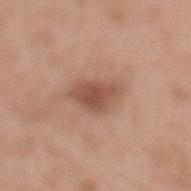{
  "biopsy_status": "not biopsied; imaged during a skin examination",
  "lesion_size": {
    "long_diameter_mm_approx": 4.5
  },
  "image": {
    "source": "total-body photography crop",
    "field_of_view_mm": 15
  },
  "patient": {
    "sex": "female",
    "age_approx": 70
  },
  "lighting": "white-light",
  "site": "mid back",
  "automated_metrics": {
    "cielab_L": 52,
    "cielab_a": 21,
    "cielab_b": 29,
    "vs_skin_darker_L": 11.0,
    "vs_skin_contrast_norm": 7.5,
    "nevus_likeness_0_100": 60
  }
}